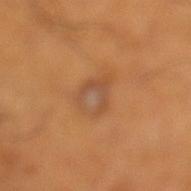Captured during whole-body skin photography for melanoma surveillance; the lesion was not biopsied. A region of skin cropped from a whole-body photographic capture, roughly 15 mm wide. A male subject approximately 55 years of age. About 3.5 mm across. From the left lower leg. The lesion-visualizer software estimated an area of roughly 7.5 mm² and a shape-asymmetry score of about 0.3 (0 = symmetric). The analysis additionally found about 6 CIELAB-L* units darker than the surrounding skin and a normalized border contrast of about 5.5. The analysis additionally found border irregularity of about 3 on a 0–10 scale and a within-lesion color-variation index near 5/10.